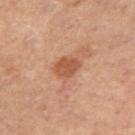The lesion was tiled from a total-body skin photograph and was not biopsied. The lesion is located on the left thigh. Automated image analysis of the tile measured an outline eccentricity of about 0.7 (0 = round, 1 = elongated). And it measured a lesion color around L≈51 a*≈24 b*≈34 in CIELAB, a lesion–skin lightness drop of about 10, and a normalized border contrast of about 7.5. A female subject aged 48–52. A 15 mm close-up tile from a total-body photography series done for melanoma screening. Imaged with cross-polarized lighting.